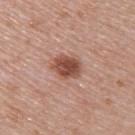Impression: Captured during whole-body skin photography for melanoma surveillance; the lesion was not biopsied. Context: The recorded lesion diameter is about 3.5 mm. Automated tile analysis of the lesion measured a footprint of about 7.5 mm² and a shape eccentricity near 0.65. The analysis additionally found an automated nevus-likeness rating near 100 out of 100 and a lesion-detection confidence of about 100/100. The patient is a female aged around 50. The lesion is on the upper back. This image is a 15 mm lesion crop taken from a total-body photograph.